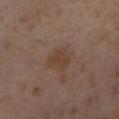Q: Was this lesion biopsied?
A: catalogued during a skin exam; not biopsied
Q: What is the imaging modality?
A: ~15 mm crop, total-body skin-cancer survey
Q: Where on the body is the lesion?
A: the right lower leg
Q: Who is the patient?
A: female, aged 53 to 57
Q: How large is the lesion?
A: ~3 mm (longest diameter)
Q: Automated lesion metrics?
A: a footprint of about 5.5 mm² and a symmetry-axis asymmetry near 0.3; a mean CIELAB color near L≈41 a*≈17 b*≈27, roughly 6 lightness units darker than nearby skin, and a lesion-to-skin contrast of about 6 (normalized; higher = more distinct); a lesion-detection confidence of about 100/100